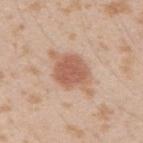The lesion was tiled from a total-body skin photograph and was not biopsied. Cropped from a whole-body photographic skin survey; the tile spans about 15 mm. A male subject, about 25 years old. Automated tile analysis of the lesion measured a shape eccentricity near 0.8. The analysis additionally found an average lesion color of about L≈59 a*≈21 b*≈31 (CIELAB), about 12 CIELAB-L* units darker than the surrounding skin, and a normalized border contrast of about 8. And it measured a within-lesion color-variation index near 2.5/10 and radial color variation of about 1. It also reported a nevus-likeness score of about 90/100 and a detector confidence of about 100 out of 100 that the crop contains a lesion. The lesion is located on the right upper arm.